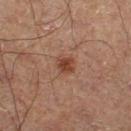Part of a total-body skin-imaging series; this lesion was reviewed on a skin check and was not flagged for biopsy. A male patient aged approximately 65. Automated tile analysis of the lesion measured an area of roughly 4.5 mm², an eccentricity of roughly 0.65, and a shape-asymmetry score of about 0.2 (0 = symmetric). It also reported a lesion color around L≈36 a*≈20 b*≈26 in CIELAB, about 8 CIELAB-L* units darker than the surrounding skin, and a normalized border contrast of about 8. It also reported a color-variation rating of about 2/10 and a peripheral color-asymmetry measure near 0.5. The software also gave a detector confidence of about 100 out of 100 that the crop contains a lesion. A lesion tile, about 15 mm wide, cut from a 3D total-body photograph. On the right lower leg.